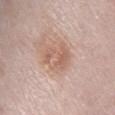notes = imaged on a skin check; not biopsied
body site = the abdomen
patient = male, aged 73–77
lesion diameter = about 4 mm
tile lighting = white-light illumination
image source = ~15 mm tile from a whole-body skin photo